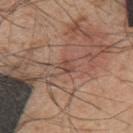<case>
<image>
  <source>total-body photography crop</source>
  <field_of_view_mm>15</field_of_view_mm>
</image>
<site>back</site>
<lesion_size>
  <long_diameter_mm_approx>2.0</long_diameter_mm_approx>
</lesion_size>
<lighting>white-light</lighting>
<automated_metrics>
  <area_mm2_approx>2.0</area_mm2_approx>
  <eccentricity>0.6</eccentricity>
  <cielab_L>46</cielab_L>
  <cielab_a>21</cielab_a>
  <cielab_b>25</cielab_b>
  <vs_skin_darker_L>7.0</vs_skin_darker_L>
  <vs_skin_contrast_norm>6.0</vs_skin_contrast_norm>
  <nevus_likeness_0_100>0</nevus_likeness_0_100>
  <lesion_detection_confidence_0_100>75</lesion_detection_confidence_0_100>
</automated_metrics>
<patient>
  <sex>male</sex>
  <age_approx>45</age_approx>
</patient>
<diagnosis>
  <histopathology>dysplastic (Clark) nevus</histopathology>
  <malignancy>benign</malignancy>
  <taxonomic_path>Benign; Benign melanocytic proliferations; Nevus; Nevus, Atypical, Dysplastic, or Clark</taxonomic_path>
</diagnosis>
</case>